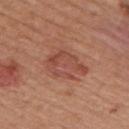Captured during whole-body skin photography for melanoma surveillance; the lesion was not biopsied.
Cropped from a whole-body photographic skin survey; the tile spans about 15 mm.
Captured under white-light illumination.
The lesion is on the back.
Automated image analysis of the tile measured a within-lesion color-variation index near 3.5/10 and a peripheral color-asymmetry measure near 1.
A male subject aged approximately 55.